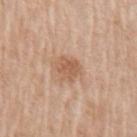Assessment:
Captured during whole-body skin photography for melanoma surveillance; the lesion was not biopsied.
Image and clinical context:
A roughly 15 mm field-of-view crop from a total-body skin photograph. From the left upper arm. Imaged with white-light lighting. The patient is a male approximately 60 years of age.